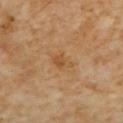Q: Was this lesion biopsied?
A: imaged on a skin check; not biopsied
Q: What are the patient's age and sex?
A: male, about 60 years old
Q: What is the imaging modality?
A: ~15 mm tile from a whole-body skin photo
Q: How large is the lesion?
A: about 2.5 mm
Q: Where on the body is the lesion?
A: the chest
Q: Automated lesion metrics?
A: an automated nevus-likeness rating near 10 out of 100 and a detector confidence of about 100 out of 100 that the crop contains a lesion
Q: How was the tile lit?
A: cross-polarized illumination